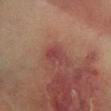Assessment: The lesion was tiled from a total-body skin photograph and was not biopsied. Acquisition and patient details: Measured at roughly 3 mm in maximum diameter. A close-up tile cropped from a whole-body skin photograph, about 15 mm across. Captured under cross-polarized illumination. From the right lower leg. A female subject aged around 70.